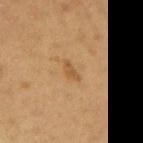biopsy status: no biopsy performed (imaged during a skin exam)
tile lighting: cross-polarized
location: the back
image: ~15 mm crop, total-body skin-cancer survey
patient: male, about 60 years old
automated metrics: a lesion area of about 2.5 mm², an eccentricity of roughly 0.9, and a shape-asymmetry score of about 0.45 (0 = symmetric); a lesion color around L≈47 a*≈18 b*≈35 in CIELAB and a normalized border contrast of about 6
size: ≈2.5 mm